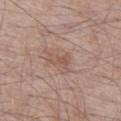The lesion was tiled from a total-body skin photograph and was not biopsied. The lesion is on the left thigh. The lesion-visualizer software estimated a border-irregularity rating of about 3/10, a color-variation rating of about 2/10, and peripheral color asymmetry of about 0.5. The software also gave a nevus-likeness score of about 5/100. This image is a 15 mm lesion crop taken from a total-body photograph. The subject is a male aged 63–67. Measured at roughly 2.5 mm in maximum diameter.